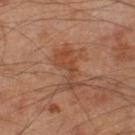follow-up=no biopsy performed (imaged during a skin exam)
diameter=about 5 mm
acquisition=15 mm crop, total-body photography
patient=male, about 65 years old
location=the right lower leg